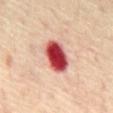subject = male, roughly 70 years of age | image = ~15 mm crop, total-body skin-cancer survey | lighting = cross-polarized illumination | lesion size = ~4.5 mm (longest diameter) | body site = the mid back.